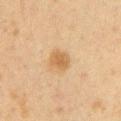Captured during whole-body skin photography for melanoma surveillance; the lesion was not biopsied.
A lesion tile, about 15 mm wide, cut from a 3D total-body photograph.
The lesion is on the left upper arm.
A female patient aged 38–42.